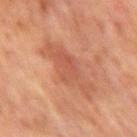Clinical impression:
The lesion was photographed on a routine skin check and not biopsied; there is no pathology result.
Image and clinical context:
Located on the right upper arm. A region of skin cropped from a whole-body photographic capture, roughly 15 mm wide. Automated image analysis of the tile measured a lesion area of about 16 mm² and a symmetry-axis asymmetry near 0.25. It also reported an automated nevus-likeness rating near 0 out of 100. A female patient, about 60 years old.